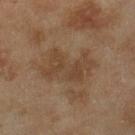No biopsy was performed on this lesion — it was imaged during a full skin examination and was not determined to be concerning. Cropped from a total-body skin-imaging series; the visible field is about 15 mm. Captured under cross-polarized illumination. Approximately 6.5 mm at its widest. Located on the right lower leg. The patient is a female aged 58–62.